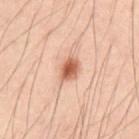Q: Is there a histopathology result?
A: no biopsy performed (imaged during a skin exam)
Q: Lesion location?
A: the abdomen
Q: Who is the patient?
A: male, roughly 40 years of age
Q: How was the tile lit?
A: cross-polarized
Q: What is the imaging modality?
A: ~15 mm crop, total-body skin-cancer survey